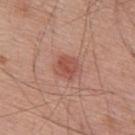No biopsy was performed on this lesion — it was imaged during a full skin examination and was not determined to be concerning.
A male patient aged 53 to 57.
An algorithmic analysis of the crop reported a nevus-likeness score of about 90/100 and a lesion-detection confidence of about 100/100.
The lesion's longest dimension is about 2.5 mm.
The lesion is on the mid back.
Cropped from a whole-body photographic skin survey; the tile spans about 15 mm.